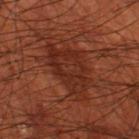The lesion was photographed on a routine skin check and not biopsied; there is no pathology result. Imaged with cross-polarized lighting. A male subject roughly 70 years of age. A close-up tile cropped from a whole-body skin photograph, about 15 mm across. Measured at roughly 7.5 mm in maximum diameter. Located on the left thigh.